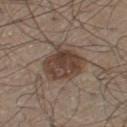Clinical impression:
Recorded during total-body skin imaging; not selected for excision or biopsy.
Acquisition and patient details:
An algorithmic analysis of the crop reported a lesion area of about 19 mm², an outline eccentricity of about 0.45 (0 = round, 1 = elongated), and a shape-asymmetry score of about 0.2 (0 = symmetric). Longest diameter approximately 5 mm. The patient is a male aged approximately 60. A close-up tile cropped from a whole-body skin photograph, about 15 mm across. On the left thigh.